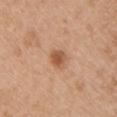Imaged during a routine full-body skin examination; the lesion was not biopsied and no histopathology is available. A male patient, aged 48 to 52. About 2.5 mm across. A close-up tile cropped from a whole-body skin photograph, about 15 mm across. This is a white-light tile. Automated tile analysis of the lesion measured a footprint of about 4 mm² and an eccentricity of roughly 0.6. The software also gave a lesion color around L≈55 a*≈23 b*≈34 in CIELAB and about 12 CIELAB-L* units darker than the surrounding skin. The analysis additionally found a border-irregularity index near 2/10, a color-variation rating of about 3/10, and a peripheral color-asymmetry measure near 1. The software also gave lesion-presence confidence of about 100/100. The lesion is on the chest.